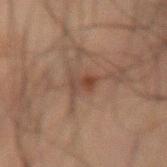Q: What is the imaging modality?
A: total-body-photography crop, ~15 mm field of view
Q: What is the anatomic site?
A: the arm
Q: What is the lesion's diameter?
A: about 3 mm
Q: Who is the patient?
A: male, aged 63–67
Q: How was the tile lit?
A: cross-polarized illumination